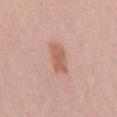workup=no biopsy performed (imaged during a skin exam); size=about 4 mm; subject=male, aged approximately 25; anatomic site=the mid back; image source=~15 mm crop, total-body skin-cancer survey; lighting=white-light.